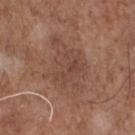biopsy status: imaged on a skin check; not biopsied
subject: male, about 70 years old
imaging modality: ~15 mm tile from a whole-body skin photo
diameter: ~6.5 mm (longest diameter)
image-analysis metrics: border irregularity of about 4.5 on a 0–10 scale, internal color variation of about 3 on a 0–10 scale, and peripheral color asymmetry of about 1; a nevus-likeness score of about 0/100
tile lighting: white-light illumination
location: the chest